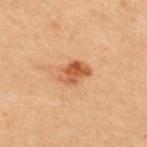Q: Was this lesion biopsied?
A: catalogued during a skin exam; not biopsied
Q: Where on the body is the lesion?
A: the upper back
Q: What kind of image is this?
A: 15 mm crop, total-body photography
Q: What are the patient's age and sex?
A: female, aged 28 to 32
Q: Lesion size?
A: ≈3.5 mm
Q: What did automated image analysis measure?
A: a border-irregularity index near 2/10 and a color-variation rating of about 6/10; a classifier nevus-likeness of about 85/100 and a lesion-detection confidence of about 100/100